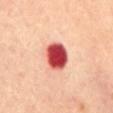biopsy status=imaged on a skin check; not biopsied.